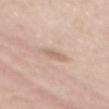<case>
<biopsy_status>not biopsied; imaged during a skin examination</biopsy_status>
<site>mid back</site>
<patient>
  <sex>female</sex>
  <age_approx>40</age_approx>
</patient>
<image>
  <source>total-body photography crop</source>
  <field_of_view_mm>15</field_of_view_mm>
</image>
<lesion_size>
  <long_diameter_mm_approx>2.5</long_diameter_mm_approx>
</lesion_size>
</case>